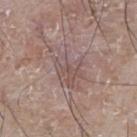Q: Is there a histopathology result?
A: imaged on a skin check; not biopsied
Q: What is the anatomic site?
A: the front of the torso
Q: Who is the patient?
A: male, about 75 years old
Q: What is the imaging modality?
A: 15 mm crop, total-body photography
Q: How large is the lesion?
A: about 6 mm